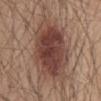patient = male, aged around 60 | imaging modality = ~15 mm tile from a whole-body skin photo | anatomic site = the mid back | lesion diameter = ≈8 mm | illumination = white-light.